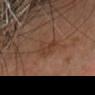The lesion was photographed on a routine skin check and not biopsied; there is no pathology result. On the head or neck. The recorded lesion diameter is about 3.5 mm. This image is a 15 mm lesion crop taken from a total-body photograph. Imaged with cross-polarized lighting. A female subject.